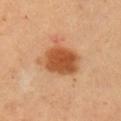notes: no biopsy performed (imaged during a skin exam); location: the right upper arm; lighting: cross-polarized illumination; automated metrics: an automated nevus-likeness rating near 100 out of 100 and lesion-presence confidence of about 100/100; size: ~5 mm (longest diameter); subject: male, about 55 years old; image source: ~15 mm crop, total-body skin-cancer survey.